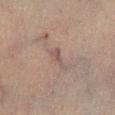Impression: This lesion was catalogued during total-body skin photography and was not selected for biopsy. Context: A close-up tile cropped from a whole-body skin photograph, about 15 mm across. Imaged with cross-polarized lighting. A male subject, approximately 75 years of age. On the left lower leg. Longest diameter approximately 3 mm.